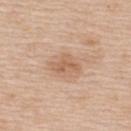| key | value |
|---|---|
| follow-up | imaged on a skin check; not biopsied |
| patient | female, aged 43 to 47 |
| image | ~15 mm tile from a whole-body skin photo |
| image-analysis metrics | a shape eccentricity near 0.75 and a shape-asymmetry score of about 0.35 (0 = symmetric); a classifier nevus-likeness of about 0/100 and a detector confidence of about 100 out of 100 that the crop contains a lesion |
| location | the upper back |
| illumination | white-light illumination |